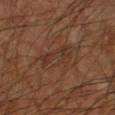| key | value |
|---|---|
| follow-up | no biopsy performed (imaged during a skin exam) |
| subject | male, about 55 years old |
| anatomic site | the left forearm |
| imaging modality | ~15 mm crop, total-body skin-cancer survey |